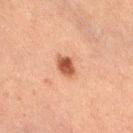The lesion was tiled from a total-body skin photograph and was not biopsied.
A female patient aged approximately 60.
This image is a 15 mm lesion crop taken from a total-body photograph.
The lesion is on the right thigh.